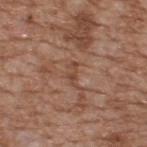workup: imaged on a skin check; not biopsied | lighting: white-light | diameter: ≈2.5 mm | subject: male, aged 63–67 | image: 15 mm crop, total-body photography | automated lesion analysis: two-axis asymmetry of about 0.3; a lesion color around L≈46 a*≈21 b*≈29 in CIELAB, roughly 7 lightness units darker than nearby skin, and a lesion-to-skin contrast of about 6 (normalized; higher = more distinct); a border-irregularity index near 5/10, a within-lesion color-variation index near 0/10, and peripheral color asymmetry of about 0 | site: the back.